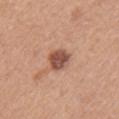| field | value |
|---|---|
| notes | catalogued during a skin exam; not biopsied |
| anatomic site | the left upper arm |
| image source | total-body-photography crop, ~15 mm field of view |
| tile lighting | white-light illumination |
| size | ~3 mm (longest diameter) |
| subject | female, about 35 years old |
| automated metrics | an area of roughly 6.5 mm², an outline eccentricity of about 0.35 (0 = round, 1 = elongated), and a shape-asymmetry score of about 0.15 (0 = symmetric); a border-irregularity rating of about 1.5/10, internal color variation of about 3.5 on a 0–10 scale, and peripheral color asymmetry of about 1.5 |